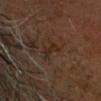Imaged during a routine full-body skin examination; the lesion was not biopsied and no histopathology is available. Cropped from a total-body skin-imaging series; the visible field is about 15 mm. Located on the head or neck. A male patient aged around 65. Longest diameter approximately 2.5 mm.